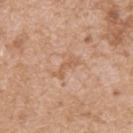notes = imaged on a skin check; not biopsied
location = the back
image = ~15 mm crop, total-body skin-cancer survey
subject = male, roughly 65 years of age